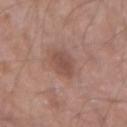Case summary:
* workup: no biopsy performed (imaged during a skin exam)
* patient: male, aged 53–57
* imaging modality: 15 mm crop, total-body photography
* location: the left forearm
* tile lighting: white-light
* diameter: ≈3 mm
* TBP lesion metrics: a mean CIELAB color near L≈49 a*≈20 b*≈25, about 9 CIELAB-L* units darker than the surrounding skin, and a lesion-to-skin contrast of about 6.5 (normalized; higher = more distinct)